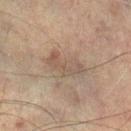Impression:
Captured during whole-body skin photography for melanoma surveillance; the lesion was not biopsied.
Image and clinical context:
The tile uses cross-polarized illumination. A region of skin cropped from a whole-body photographic capture, roughly 15 mm wide. On the right lower leg. Automated image analysis of the tile measured a lesion color around L≈45 a*≈12 b*≈22 in CIELAB, a lesion–skin lightness drop of about 6, and a lesion-to-skin contrast of about 5.5 (normalized; higher = more distinct). The subject is a male about 75 years old. Longest diameter approximately 5 mm.